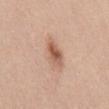Assessment:
Imaged during a routine full-body skin examination; the lesion was not biopsied and no histopathology is available.
Context:
A 15 mm close-up extracted from a 3D total-body photography capture. A male subject about 60 years old. Automated tile analysis of the lesion measured border irregularity of about 2 on a 0–10 scale, internal color variation of about 5.5 on a 0–10 scale, and a peripheral color-asymmetry measure near 2. The software also gave a nevus-likeness score of about 95/100 and a detector confidence of about 100 out of 100 that the crop contains a lesion. The lesion is on the mid back.